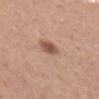No biopsy was performed on this lesion — it was imaged during a full skin examination and was not determined to be concerning. A 15 mm close-up extracted from a 3D total-body photography capture. A female patient, in their 30s. The recorded lesion diameter is about 4 mm. On the left forearm. The tile uses white-light illumination.